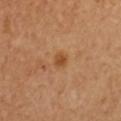Clinical impression:
Recorded during total-body skin imaging; not selected for excision or biopsy.
Acquisition and patient details:
Longest diameter approximately 2 mm. Cropped from a whole-body photographic skin survey; the tile spans about 15 mm. A female subject, aged approximately 50. Located on the chest. Imaged with cross-polarized lighting.